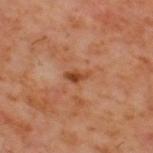follow-up: no biopsy performed (imaged during a skin exam) | size: ≈2.5 mm | location: the upper back | tile lighting: cross-polarized illumination | patient: male, aged 58 to 62 | acquisition: 15 mm crop, total-body photography.